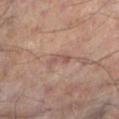This lesion was catalogued during total-body skin photography and was not selected for biopsy. The lesion is on the left lower leg. Automated image analysis of the tile measured an average lesion color of about L≈49 a*≈19 b*≈22 (CIELAB) and roughly 7 lightness units darker than nearby skin. And it measured a border-irregularity index near 5.5/10, a within-lesion color-variation index near 0/10, and a peripheral color-asymmetry measure near 0. The patient is a male aged approximately 55. A 15 mm crop from a total-body photograph taken for skin-cancer surveillance. Longest diameter approximately 3 mm. The tile uses cross-polarized illumination.